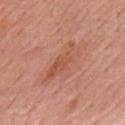biopsy status = catalogued during a skin exam; not biopsied | acquisition = ~15 mm tile from a whole-body skin photo | lighting = white-light illumination | anatomic site = the mid back | subject = male, aged 78 to 82 | diameter = ~5.5 mm (longest diameter).